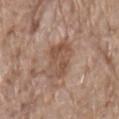Imaged during a routine full-body skin examination; the lesion was not biopsied and no histopathology is available.
Approximately 5 mm at its widest.
A lesion tile, about 15 mm wide, cut from a 3D total-body photograph.
On the lower back.
Captured under white-light illumination.
A male patient, aged 78–82.
Automated image analysis of the tile measured a border-irregularity rating of about 2.5/10, a color-variation rating of about 4/10, and radial color variation of about 1.5. The software also gave a detector confidence of about 100 out of 100 that the crop contains a lesion.